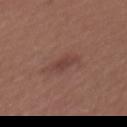The lesion was photographed on a routine skin check and not biopsied; there is no pathology result. Measured at roughly 4.5 mm in maximum diameter. Automated image analysis of the tile measured a lesion area of about 6 mm², an outline eccentricity of about 0.95 (0 = round, 1 = elongated), and a symmetry-axis asymmetry near 0.25. The software also gave a lesion color around L≈42 a*≈21 b*≈23 in CIELAB, roughly 7 lightness units darker than nearby skin, and a normalized lesion–skin contrast near 6. It also reported a classifier nevus-likeness of about 5/100 and lesion-presence confidence of about 100/100. A close-up tile cropped from a whole-body skin photograph, about 15 mm across. The patient is a female in their mid-20s. The tile uses white-light illumination. From the mid back.